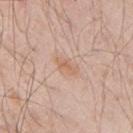workup: imaged on a skin check; not biopsied
image source: total-body-photography crop, ~15 mm field of view
anatomic site: the right upper arm
subject: male, aged 43 to 47
illumination: white-light illumination
lesion size: ≈2.5 mm
automated lesion analysis: a lesion color around L≈62 a*≈20 b*≈32 in CIELAB, a lesion–skin lightness drop of about 7, and a normalized border contrast of about 6